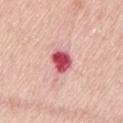Assessment: No biopsy was performed on this lesion — it was imaged during a full skin examination and was not determined to be concerning. Acquisition and patient details: The lesion is located on the left thigh. This is a white-light tile. A lesion tile, about 15 mm wide, cut from a 3D total-body photograph. About 3 mm across. The patient is a male aged 78–82.